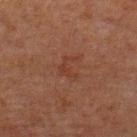Clinical impression: The lesion was tiled from a total-body skin photograph and was not biopsied.